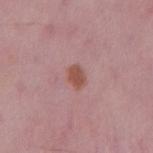Imaged during a routine full-body skin examination; the lesion was not biopsied and no histopathology is available. Imaged with white-light lighting. A male patient, aged approximately 50. Located on the mid back. The total-body-photography lesion software estimated an area of roughly 4 mm², a shape eccentricity near 0.7, and a shape-asymmetry score of about 0.25 (0 = symmetric). The software also gave a border-irregularity rating of about 2/10 and internal color variation of about 1.5 on a 0–10 scale. The analysis additionally found a detector confidence of about 100 out of 100 that the crop contains a lesion. This image is a 15 mm lesion crop taken from a total-body photograph.